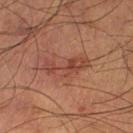Imaged during a routine full-body skin examination; the lesion was not biopsied and no histopathology is available.
The subject is a male aged approximately 55.
About 5.5 mm across.
A roughly 15 mm field-of-view crop from a total-body skin photograph.
From the leg.
Automated image analysis of the tile measured a lesion area of about 8.5 mm², a shape eccentricity near 0.95, and a shape-asymmetry score of about 0.4 (0 = symmetric). And it measured a mean CIELAB color near L≈40 a*≈23 b*≈27, a lesion–skin lightness drop of about 8, and a lesion-to-skin contrast of about 6.5 (normalized; higher = more distinct). The software also gave a border-irregularity index near 6.5/10, a color-variation rating of about 3.5/10, and radial color variation of about 1.
This is a cross-polarized tile.